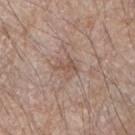notes = imaged on a skin check; not biopsied
imaging modality = total-body-photography crop, ~15 mm field of view
lesion diameter = about 2.5 mm
TBP lesion metrics = a mean CIELAB color near L≈53 a*≈17 b*≈26 and a normalized lesion–skin contrast near 5.5; a border-irregularity index near 6/10 and internal color variation of about 1 on a 0–10 scale; a nevus-likeness score of about 0/100
anatomic site = the right forearm
patient = male, roughly 75 years of age